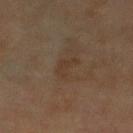notes: no biopsy performed (imaged during a skin exam)
subject: female, in their mid- to late 50s
image: ~15 mm crop, total-body skin-cancer survey
body site: the left lower leg